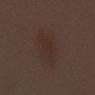Assessment:
Imaged during a routine full-body skin examination; the lesion was not biopsied and no histopathology is available.
Clinical summary:
A roughly 15 mm field-of-view crop from a total-body skin photograph. The patient is a female aged 48 to 52. An algorithmic analysis of the crop reported an outline eccentricity of about 0.85 (0 = round, 1 = elongated). The software also gave an average lesion color of about L≈28 a*≈14 b*≈20 (CIELAB) and about 4 CIELAB-L* units darker than the surrounding skin. And it measured a border-irregularity rating of about 2.5/10, internal color variation of about 2 on a 0–10 scale, and a peripheral color-asymmetry measure near 1. It also reported a classifier nevus-likeness of about 15/100. About 5.5 mm across. The lesion is located on the abdomen. The tile uses white-light illumination.